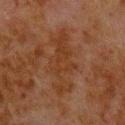Recorded during total-body skin imaging; not selected for excision or biopsy. Longest diameter approximately 8 mm. A male patient, approximately 80 years of age. The tile uses cross-polarized illumination. The total-body-photography lesion software estimated a lesion area of about 16 mm², an outline eccentricity of about 0.95 (0 = round, 1 = elongated), and a symmetry-axis asymmetry near 0.45. The analysis additionally found a nevus-likeness score of about 0/100 and a detector confidence of about 80 out of 100 that the crop contains a lesion. Located on the upper back. Cropped from a total-body skin-imaging series; the visible field is about 15 mm.